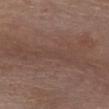| key | value |
|---|---|
| workup | total-body-photography surveillance lesion; no biopsy |
| image source | 15 mm crop, total-body photography |
| site | the chest |
| subject | male, in their mid- to late 70s |